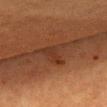| feature | finding |
|---|---|
| biopsy status | imaged on a skin check; not biopsied |